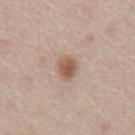Image and clinical context: From the abdomen. A roughly 15 mm field-of-view crop from a total-body skin photograph. The lesion-visualizer software estimated a footprint of about 5 mm² and an outline eccentricity of about 0.65 (0 = round, 1 = elongated). It also reported an average lesion color of about L≈56 a*≈18 b*≈28 (CIELAB). A male subject in their mid-60s. The tile uses white-light illumination. About 3 mm across.